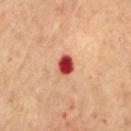No biopsy was performed on this lesion — it was imaged during a full skin examination and was not determined to be concerning.
This is a cross-polarized tile.
Automated image analysis of the tile measured border irregularity of about 1 on a 0–10 scale and peripheral color asymmetry of about 1.5.
Longest diameter approximately 2.5 mm.
The lesion is located on the back.
A 15 mm close-up tile from a total-body photography series done for melanoma screening.
A male subject, aged 63–67.